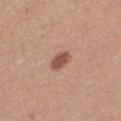The lesion was photographed on a routine skin check and not biopsied; there is no pathology result. A 15 mm close-up extracted from a 3D total-body photography capture. Longest diameter approximately 2.5 mm. The lesion-visualizer software estimated an average lesion color of about L≈52 a*≈22 b*≈29 (CIELAB), roughly 13 lightness units darker than nearby skin, and a lesion-to-skin contrast of about 9 (normalized; higher = more distinct). The analysis additionally found a peripheral color-asymmetry measure near 0.5. And it measured a nevus-likeness score of about 90/100 and a lesion-detection confidence of about 100/100. The tile uses white-light illumination. On the left thigh. A female subject in their mid-30s.